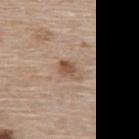{"biopsy_status": "not biopsied; imaged during a skin examination", "site": "upper back", "image": {"source": "total-body photography crop", "field_of_view_mm": 15}, "patient": {"sex": "female", "age_approx": 65}}